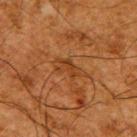Captured during whole-body skin photography for melanoma surveillance; the lesion was not biopsied. The lesion's longest dimension is about 4 mm. Imaged with cross-polarized lighting. Cropped from a whole-body photographic skin survey; the tile spans about 15 mm. A male subject about 65 years old. On the back. Automated tile analysis of the lesion measured a nevus-likeness score of about 0/100 and a detector confidence of about 80 out of 100 that the crop contains a lesion.